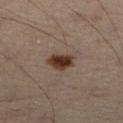follow-up = total-body-photography surveillance lesion; no biopsy
site = the left leg
imaging modality = total-body-photography crop, ~15 mm field of view
automated lesion analysis = border irregularity of about 2 on a 0–10 scale, a within-lesion color-variation index near 4/10, and radial color variation of about 1; a classifier nevus-likeness of about 100/100 and a detector confidence of about 100 out of 100 that the crop contains a lesion
patient = male, roughly 50 years of age
tile lighting = cross-polarized illumination